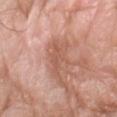follow-up: total-body-photography surveillance lesion; no biopsy
subject: male, aged 58–62
TBP lesion metrics: an area of roughly 8.5 mm², an outline eccentricity of about 0.8 (0 = round, 1 = elongated), and a symmetry-axis asymmetry near 0.35; a nevus-likeness score of about 0/100 and a lesion-detection confidence of about 95/100
acquisition: total-body-photography crop, ~15 mm field of view
anatomic site: the left forearm
tile lighting: white-light illumination